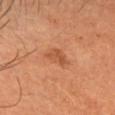biopsy status: total-body-photography surveillance lesion; no biopsy
automated lesion analysis: a lesion area of about 5 mm²; a mean CIELAB color near L≈52 a*≈26 b*≈37, roughly 8 lightness units darker than nearby skin, and a normalized lesion–skin contrast near 5.5; border irregularity of about 3 on a 0–10 scale, a within-lesion color-variation index near 2.5/10, and a peripheral color-asymmetry measure near 1; a nevus-likeness score of about 25/100
size: ~3 mm (longest diameter)
imaging modality: ~15 mm tile from a whole-body skin photo
location: the head or neck
illumination: cross-polarized illumination
patient: female, approximately 65 years of age